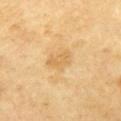Q: Was a biopsy performed?
A: imaged on a skin check; not biopsied
Q: What is the imaging modality?
A: ~15 mm crop, total-body skin-cancer survey
Q: What is the anatomic site?
A: the left upper arm
Q: Illumination type?
A: cross-polarized illumination
Q: Automated lesion metrics?
A: an area of roughly 5.5 mm², an outline eccentricity of about 0.7 (0 = round, 1 = elongated), and two-axis asymmetry of about 0.3; a border-irregularity rating of about 3.5/10 and peripheral color asymmetry of about 0.5
Q: Lesion size?
A: about 3 mm
Q: Patient demographics?
A: male, aged approximately 70